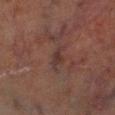Captured during whole-body skin photography for melanoma surveillance; the lesion was not biopsied. A 15 mm crop from a total-body photograph taken for skin-cancer surveillance. Longest diameter approximately 3 mm. Captured under cross-polarized illumination. The lesion is located on the left lower leg. A male patient roughly 70 years of age.